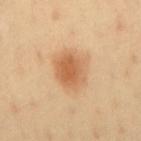Q: Was a biopsy performed?
A: no biopsy performed (imaged during a skin exam)
Q: How large is the lesion?
A: ≈4 mm
Q: Who is the patient?
A: male, about 40 years old
Q: What lighting was used for the tile?
A: cross-polarized
Q: What is the imaging modality?
A: ~15 mm crop, total-body skin-cancer survey
Q: What is the anatomic site?
A: the back